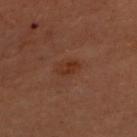Recorded during total-body skin imaging; not selected for excision or biopsy. From the chest. Longest diameter approximately 2.5 mm. Captured under cross-polarized illumination. The subject is a female roughly 50 years of age. A region of skin cropped from a whole-body photographic capture, roughly 15 mm wide.